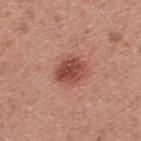Impression:
This lesion was catalogued during total-body skin photography and was not selected for biopsy.
Acquisition and patient details:
A male subject, aged around 30. A region of skin cropped from a whole-body photographic capture, roughly 15 mm wide. This is a white-light tile. The lesion is on the upper back. The recorded lesion diameter is about 3.5 mm.